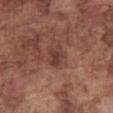| field | value |
|---|---|
| biopsy status | catalogued during a skin exam; not biopsied |